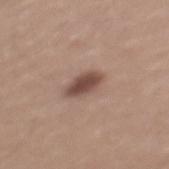This lesion was catalogued during total-body skin photography and was not selected for biopsy.